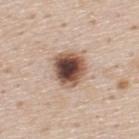Q: What is the lesion's diameter?
A: about 4 mm
Q: What lighting was used for the tile?
A: white-light illumination
Q: How was this image acquired?
A: 15 mm crop, total-body photography
Q: Patient demographics?
A: male, aged 43 to 47
Q: Lesion location?
A: the upper back